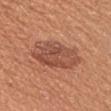Q: Who is the patient?
A: male, approximately 45 years of age
Q: Illumination type?
A: white-light illumination
Q: What is the imaging modality?
A: 15 mm crop, total-body photography
Q: What did automated image analysis measure?
A: a border-irregularity rating of about 2.5/10 and internal color variation of about 4.5 on a 0–10 scale
Q: What is the anatomic site?
A: the chest
Q: Lesion size?
A: ~6 mm (longest diameter)